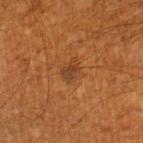Case summary:
* workup — imaged on a skin check; not biopsied
* body site — the right lower leg
* subject — male, aged 58 to 62
* TBP lesion metrics — an eccentricity of roughly 0.65 and a symmetry-axis asymmetry near 0.35
* illumination — cross-polarized
* lesion size — about 2.5 mm
* image source — 15 mm crop, total-body photography